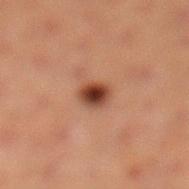The lesion was tiled from a total-body skin photograph and was not biopsied. About 2.5 mm across. The lesion is located on the left lower leg. A female subject, aged approximately 40. Captured under cross-polarized illumination. The total-body-photography lesion software estimated a border-irregularity rating of about 1.5/10, a within-lesion color-variation index near 5.5/10, and radial color variation of about 1.5. A 15 mm crop from a total-body photograph taken for skin-cancer surveillance.